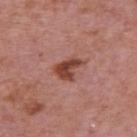image-analysis metrics: an outline eccentricity of about 0.75 (0 = round, 1 = elongated) and a shape-asymmetry score of about 0.4 (0 = symmetric); a mean CIELAB color near L≈44 a*≈26 b*≈27, a lesion–skin lightness drop of about 13, and a normalized border contrast of about 9.5
acquisition: total-body-photography crop, ~15 mm field of view
patient: male, aged around 75
site: the upper back
lesion size: about 4 mm
tile lighting: white-light illumination